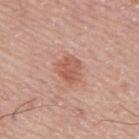Assessment:
The lesion was tiled from a total-body skin photograph and was not biopsied.
Clinical summary:
The subject is a male aged 73–77. Approximately 3 mm at its widest. The lesion is located on the mid back. This image is a 15 mm lesion crop taken from a total-body photograph.